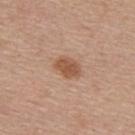Automated tile analysis of the lesion measured a lesion area of about 6 mm², an outline eccentricity of about 0.75 (0 = round, 1 = elongated), and a shape-asymmetry score of about 0.2 (0 = symmetric). The analysis additionally found a normalized border contrast of about 8. The tile uses white-light illumination. The lesion is located on the mid back. Cropped from a whole-body photographic skin survey; the tile spans about 15 mm. The recorded lesion diameter is about 3 mm. A male subject approximately 55 years of age.